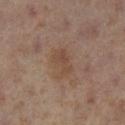{
  "biopsy_status": "not biopsied; imaged during a skin examination",
  "automated_metrics": {
    "cielab_L": 45,
    "cielab_a": 17,
    "cielab_b": 27,
    "vs_skin_darker_L": 6.0,
    "vs_skin_contrast_norm": 5.5,
    "border_irregularity_0_10": 4.0,
    "color_variation_0_10": 3.5,
    "peripheral_color_asymmetry": 1.0,
    "nevus_likeness_0_100": 0
  },
  "site": "leg",
  "patient": {
    "sex": "female",
    "age_approx": 40
  },
  "lighting": "cross-polarized",
  "lesion_size": {
    "long_diameter_mm_approx": 3.5
  },
  "image": {
    "source": "total-body photography crop",
    "field_of_view_mm": 15
  }
}